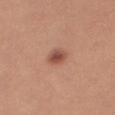Recorded during total-body skin imaging; not selected for excision or biopsy. From the right thigh. A region of skin cropped from a whole-body photographic capture, roughly 15 mm wide. The patient is a female aged around 25.